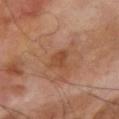| field | value |
|---|---|
| notes | total-body-photography surveillance lesion; no biopsy |
| image | ~15 mm tile from a whole-body skin photo |
| patient | male, roughly 70 years of age |
| anatomic site | the right upper arm |